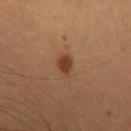Q: Was this lesion biopsied?
A: total-body-photography surveillance lesion; no biopsy
Q: Patient demographics?
A: male, aged approximately 65
Q: How was this image acquired?
A: ~15 mm tile from a whole-body skin photo
Q: What is the anatomic site?
A: the mid back
Q: How was the tile lit?
A: cross-polarized illumination
Q: How large is the lesion?
A: about 3 mm
Q: What did automated image analysis measure?
A: an area of roughly 4.5 mm², an eccentricity of roughly 0.8, and a symmetry-axis asymmetry near 0.25; border irregularity of about 2.5 on a 0–10 scale, a color-variation rating of about 2/10, and a peripheral color-asymmetry measure near 0.5; a detector confidence of about 100 out of 100 that the crop contains a lesion